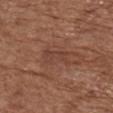notes = total-body-photography surveillance lesion; no biopsy | location = the front of the torso | lesion diameter = ~3.5 mm (longest diameter) | image = total-body-photography crop, ~15 mm field of view | tile lighting = white-light | patient = female, aged 73–77.